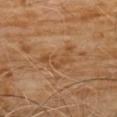Impression:
Recorded during total-body skin imaging; not selected for excision or biopsy.
Acquisition and patient details:
A 15 mm close-up extracted from a 3D total-body photography capture. Automated image analysis of the tile measured a lesion color around L≈46 a*≈20 b*≈35 in CIELAB, roughly 7 lightness units darker than nearby skin, and a normalized border contrast of about 5.5. The lesion is on the chest. Captured under cross-polarized illumination. A male patient in their 60s.